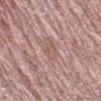Q: Was this lesion biopsied?
A: no biopsy performed (imaged during a skin exam)
Q: Lesion location?
A: the right forearm
Q: How large is the lesion?
A: about 2.5 mm
Q: What did automated image analysis measure?
A: an area of roughly 3.5 mm², an eccentricity of roughly 0.8, and a shape-asymmetry score of about 0.25 (0 = symmetric); a border-irregularity rating of about 2.5/10, a color-variation rating of about 2/10, and radial color variation of about 0.5; a nevus-likeness score of about 0/100
Q: What kind of image is this?
A: ~15 mm crop, total-body skin-cancer survey
Q: Patient demographics?
A: male, in their mid- to late 60s